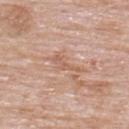workup=catalogued during a skin exam; not biopsied | location=the upper back | subject=male, about 75 years old | illumination=white-light illumination | acquisition=~15 mm tile from a whole-body skin photo.